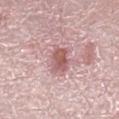Assessment:
The lesion was tiled from a total-body skin photograph and was not biopsied.